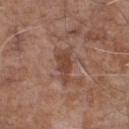Q: What is the imaging modality?
A: ~15 mm tile from a whole-body skin photo
Q: Where on the body is the lesion?
A: the chest
Q: What are the patient's age and sex?
A: male, aged 68–72